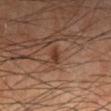Notes:
– workup · no biopsy performed (imaged during a skin exam)
– image source · 15 mm crop, total-body photography
– tile lighting · cross-polarized illumination
– size · about 2 mm
– patient · male, aged 63–67
– site · the left lower leg